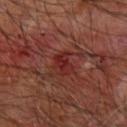Findings:
* notes: total-body-photography surveillance lesion; no biopsy
* tile lighting: cross-polarized illumination
* image source: 15 mm crop, total-body photography
* body site: the arm
* subject: male, approximately 65 years of age
* image-analysis metrics: a mean CIELAB color near L≈27 a*≈29 b*≈24, roughly 7 lightness units darker than nearby skin, and a normalized border contrast of about 7.5; a border-irregularity rating of about 3.5/10; a classifier nevus-likeness of about 0/100 and lesion-presence confidence of about 100/100
* diameter: ≈2.5 mm